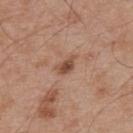Case summary:
– notes · no biopsy performed (imaged during a skin exam)
– image source · total-body-photography crop, ~15 mm field of view
– anatomic site · the upper back
– subject · male, in their mid- to late 50s